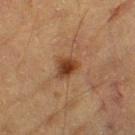workup: no biopsy performed (imaged during a skin exam)
size: ≈2.5 mm
patient: male, about 85 years old or older
illumination: cross-polarized
anatomic site: the left thigh
image: total-body-photography crop, ~15 mm field of view